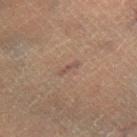A male patient roughly 50 years of age.
A 15 mm close-up extracted from a 3D total-body photography capture.
Imaged with cross-polarized lighting.
On the right lower leg.